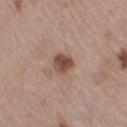Part of a total-body skin-imaging series; this lesion was reviewed on a skin check and was not flagged for biopsy.
The recorded lesion diameter is about 3 mm.
Cropped from a whole-body photographic skin survey; the tile spans about 15 mm.
From the left thigh.
The total-body-photography lesion software estimated an area of roughly 5.5 mm², a shape eccentricity near 0.65, and two-axis asymmetry of about 0.25. It also reported an average lesion color of about L≈48 a*≈19 b*≈27 (CIELAB), a lesion–skin lightness drop of about 13, and a normalized lesion–skin contrast near 9.5. The software also gave a border-irregularity index near 2/10, a within-lesion color-variation index near 3.5/10, and a peripheral color-asymmetry measure near 1. It also reported an automated nevus-likeness rating near 90 out of 100.
This is a white-light tile.
A female patient in their 40s.